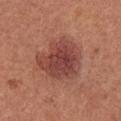Impression:
Captured during whole-body skin photography for melanoma surveillance; the lesion was not biopsied.
Context:
A 15 mm close-up extracted from a 3D total-body photography capture. From the upper back. A female subject, in their mid- to late 30s. The total-body-photography lesion software estimated an area of roughly 23 mm² and a shape-asymmetry score of about 0.25 (0 = symmetric). The analysis additionally found an average lesion color of about L≈45 a*≈26 b*≈26 (CIELAB) and a normalized lesion–skin contrast near 8.5. It also reported border irregularity of about 3 on a 0–10 scale and a color-variation rating of about 4.5/10. Longest diameter approximately 5.5 mm. Imaged with white-light lighting.